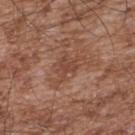No biopsy was performed on this lesion — it was imaged during a full skin examination and was not determined to be concerning. Approximately 4.5 mm at its widest. A 15 mm crop from a total-body photograph taken for skin-cancer surveillance. A male patient aged approximately 55. Captured under white-light illumination. The lesion is on the back.